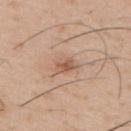Imaged with white-light lighting.
About 3 mm across.
A 15 mm close-up extracted from a 3D total-body photography capture.
Automated image analysis of the tile measured two-axis asymmetry of about 0.3. And it measured a nevus-likeness score of about 20/100 and a lesion-detection confidence of about 100/100.
A male patient aged approximately 55.
The lesion is on the right upper arm.